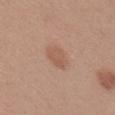| feature | finding |
|---|---|
| follow-up | imaged on a skin check; not biopsied |
| automated metrics | a footprint of about 5.5 mm², an outline eccentricity of about 0.75 (0 = round, 1 = elongated), and two-axis asymmetry of about 0.2; a normalized border contrast of about 5; a lesion-detection confidence of about 100/100 |
| subject | female, aged approximately 35 |
| acquisition | ~15 mm crop, total-body skin-cancer survey |
| lesion diameter | ~3 mm (longest diameter) |
| site | the head or neck |